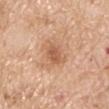Recorded during total-body skin imaging; not selected for excision or biopsy.
Captured under white-light illumination.
The lesion's longest dimension is about 2.5 mm.
A male subject, aged 58 to 62.
From the right upper arm.
Cropped from a whole-body photographic skin survey; the tile spans about 15 mm.